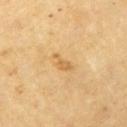Imaged during a routine full-body skin examination; the lesion was not biopsied and no histopathology is available. Located on the left upper arm. A 15 mm close-up tile from a total-body photography series done for melanoma screening. A male subject about 70 years old. Captured under cross-polarized illumination. About 3 mm across. Automated tile analysis of the lesion measured a lesion area of about 3 mm², an eccentricity of roughly 0.9, and a shape-asymmetry score of about 0.55 (0 = symmetric). The analysis additionally found a lesion–skin lightness drop of about 8 and a normalized border contrast of about 5.5. It also reported an automated nevus-likeness rating near 0 out of 100.